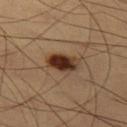biopsy status — imaged on a skin check; not biopsied | patient — male, aged 58–62 | lesion diameter — about 4 mm | image — 15 mm crop, total-body photography | body site — the left thigh | illumination — cross-polarized illumination.